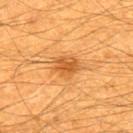The lesion was photographed on a routine skin check and not biopsied; there is no pathology result. A male subject about 60 years old. Cropped from a whole-body photographic skin survey; the tile spans about 15 mm. Approximately 3.5 mm at its widest. An algorithmic analysis of the crop reported a border-irregularity rating of about 2.5/10, a within-lesion color-variation index near 3/10, and peripheral color asymmetry of about 1. From the upper back. Imaged with cross-polarized lighting.